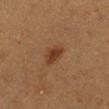Imaged during a routine full-body skin examination; the lesion was not biopsied and no histopathology is available. Measured at roughly 3.5 mm in maximum diameter. The lesion is located on the left lower leg. A female subject in their 40s. A 15 mm close-up extracted from a 3D total-body photography capture.